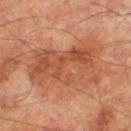The lesion was photographed on a routine skin check and not biopsied; there is no pathology result.
The lesion-visualizer software estimated an area of roughly 34 mm² and a shape-asymmetry score of about 0.25 (0 = symmetric). The software also gave an average lesion color of about L≈51 a*≈27 b*≈35 (CIELAB) and about 9 CIELAB-L* units darker than the surrounding skin. And it measured a border-irregularity index near 5/10, a within-lesion color-variation index near 6/10, and a peripheral color-asymmetry measure near 2. The analysis additionally found a classifier nevus-likeness of about 0/100 and lesion-presence confidence of about 100/100.
The tile uses cross-polarized illumination.
The lesion is on the right lower leg.
A region of skin cropped from a whole-body photographic capture, roughly 15 mm wide.
A male subject aged approximately 70.